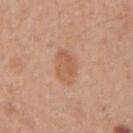Part of a total-body skin-imaging series; this lesion was reviewed on a skin check and was not flagged for biopsy. Automated tile analysis of the lesion measured an area of roughly 8.5 mm², an eccentricity of roughly 0.55, and a shape-asymmetry score of about 0.2 (0 = symmetric). It also reported a classifier nevus-likeness of about 15/100. The lesion is located on the left forearm. Cropped from a whole-body photographic skin survey; the tile spans about 15 mm. A female patient about 35 years old.